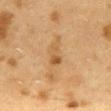{
  "image": {
    "source": "total-body photography crop",
    "field_of_view_mm": 15
  },
  "patient": {
    "sex": "female",
    "age_approx": 40
  },
  "site": "mid back",
  "lesion_size": {
    "long_diameter_mm_approx": 3.0
  }
}